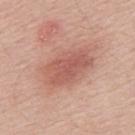imaging modality = 15 mm crop, total-body photography | subject = male, approximately 55 years of age | body site = the upper back.